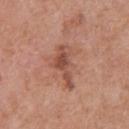Part of a total-body skin-imaging series; this lesion was reviewed on a skin check and was not flagged for biopsy. Automated image analysis of the tile measured roughly 11 lightness units darker than nearby skin and a normalized border contrast of about 7.5. A 15 mm close-up extracted from a 3D total-body photography capture. A male patient, about 70 years old. This is a white-light tile. On the front of the torso. The lesion's longest dimension is about 5.5 mm.